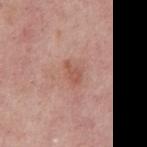Imaged during a routine full-body skin examination; the lesion was not biopsied and no histopathology is available.
From the mid back.
This is a white-light tile.
About 2.5 mm across.
Automated tile analysis of the lesion measured a lesion area of about 3.5 mm².
A close-up tile cropped from a whole-body skin photograph, about 15 mm across.
A male subject, in their mid-50s.